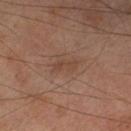Impression: The lesion was photographed on a routine skin check and not biopsied; there is no pathology result. Image and clinical context: A male subject aged around 65. Cropped from a whole-body photographic skin survey; the tile spans about 15 mm. The lesion is on the left thigh.